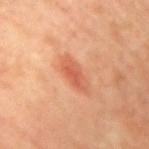- notes: catalogued during a skin exam; not biopsied
- lesion diameter: about 4 mm
- subject: female, approximately 65 years of age
- location: the arm
- imaging modality: total-body-photography crop, ~15 mm field of view
- lighting: cross-polarized